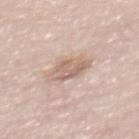Findings:
• biopsy status: imaged on a skin check; not biopsied